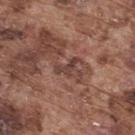Q: Was this lesion biopsied?
A: no biopsy performed (imaged during a skin exam)
Q: Automated lesion metrics?
A: a lesion color around L≈40 a*≈20 b*≈22 in CIELAB and roughly 9 lightness units darker than nearby skin; a within-lesion color-variation index near 0/10 and peripheral color asymmetry of about 0; an automated nevus-likeness rating near 0 out of 100 and a lesion-detection confidence of about 75/100
Q: What is the imaging modality?
A: 15 mm crop, total-body photography
Q: How large is the lesion?
A: about 3 mm
Q: What lighting was used for the tile?
A: white-light illumination
Q: Lesion location?
A: the upper back
Q: Patient demographics?
A: male, in their mid-70s